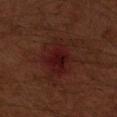biopsy status = catalogued during a skin exam; not biopsied | patient = male, about 60 years old | location = the right upper arm | image = 15 mm crop, total-body photography.